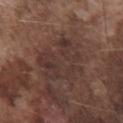• workup: imaged on a skin check; not biopsied
• body site: the chest
• subject: male, aged 73 to 77
• tile lighting: white-light illumination
• automated metrics: a footprint of about 21 mm², an eccentricity of roughly 0.45, and two-axis asymmetry of about 0.3; roughly 6 lightness units darker than nearby skin and a normalized border contrast of about 6; a border-irregularity rating of about 4.5/10 and radial color variation of about 1
• lesion size: ~5.5 mm (longest diameter)
• image: total-body-photography crop, ~15 mm field of view